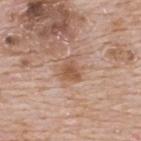biopsy status = catalogued during a skin exam; not biopsied | tile lighting = white-light illumination | site = the upper back | lesion diameter = ≈2.5 mm | automated lesion analysis = a lesion area of about 5 mm², an eccentricity of roughly 0.6, and two-axis asymmetry of about 0.35; a classifier nevus-likeness of about 10/100 and a detector confidence of about 100 out of 100 that the crop contains a lesion | patient = male, aged around 65 | image source = total-body-photography crop, ~15 mm field of view.